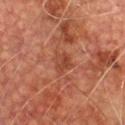Clinical impression:
Part of a total-body skin-imaging series; this lesion was reviewed on a skin check and was not flagged for biopsy.
Background:
A lesion tile, about 15 mm wide, cut from a 3D total-body photograph. A male patient aged 73 to 77. On the chest. This is a cross-polarized tile. Automated image analysis of the tile measured a shape-asymmetry score of about 0.35 (0 = symmetric). It also reported a classifier nevus-likeness of about 0/100 and lesion-presence confidence of about 100/100. Measured at roughly 3 mm in maximum diameter.